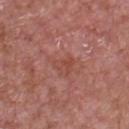On the chest. Cropped from a whole-body photographic skin survey; the tile spans about 15 mm. The patient is a male aged approximately 65. Imaged with white-light lighting. An algorithmic analysis of the crop reported an area of roughly 3.5 mm² and two-axis asymmetry of about 0.4. And it measured a normalized lesion–skin contrast near 5.5. It also reported border irregularity of about 4.5 on a 0–10 scale, a within-lesion color-variation index near 0/10, and radial color variation of about 0. The analysis additionally found an automated nevus-likeness rating near 0 out of 100. About 2.5 mm across.